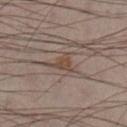This lesion was catalogued during total-body skin photography and was not selected for biopsy.
A lesion tile, about 15 mm wide, cut from a 3D total-body photograph.
The subject is a male roughly 40 years of age.
This is a cross-polarized tile.
The total-body-photography lesion software estimated a footprint of about 5 mm² and a shape-asymmetry score of about 0.55 (0 = symmetric).
From the right lower leg.
Measured at roughly 4 mm in maximum diameter.